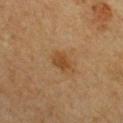From the chest. A female patient, aged 38–42. A lesion tile, about 15 mm wide, cut from a 3D total-body photograph.